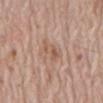Q: Is there a histopathology result?
A: total-body-photography surveillance lesion; no biopsy
Q: What did automated image analysis measure?
A: a nevus-likeness score of about 0/100 and a lesion-detection confidence of about 100/100
Q: What is the imaging modality?
A: total-body-photography crop, ~15 mm field of view
Q: Who is the patient?
A: male, approximately 70 years of age
Q: Illumination type?
A: white-light illumination
Q: Where on the body is the lesion?
A: the abdomen